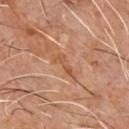Findings:
- biopsy status — imaged on a skin check; not biopsied
- patient — male, aged around 60
- lesion size — about 4 mm
- lighting — cross-polarized illumination
- acquisition — total-body-photography crop, ~15 mm field of view
- site — the front of the torso